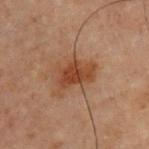The total-body-photography lesion software estimated an area of roughly 11 mm², a shape eccentricity near 0.75, and a symmetry-axis asymmetry near 0.4. It also reported about 8 CIELAB-L* units darker than the surrounding skin and a normalized border contrast of about 8.
The tile uses cross-polarized illumination.
A male subject, aged approximately 70.
Approximately 5 mm at its widest.
A roughly 15 mm field-of-view crop from a total-body skin photograph.
The lesion is located on the chest.